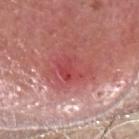The lesion was photographed on a routine skin check and not biopsied; there is no pathology result. The total-body-photography lesion software estimated a mean CIELAB color near L≈50 a*≈35 b*≈25, about 9 CIELAB-L* units darker than the surrounding skin, and a normalized lesion–skin contrast near 6. The analysis additionally found a border-irregularity rating of about 4/10 and a within-lesion color-variation index near 5/10. About 4.5 mm across. From the head or neck. A male patient approximately 55 years of age. A close-up tile cropped from a whole-body skin photograph, about 15 mm across.